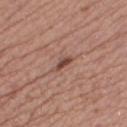Recorded during total-body skin imaging; not selected for excision or biopsy. Approximately 3 mm at its widest. A female patient, aged 38–42. Located on the right lower leg. Automated image analysis of the tile measured an eccentricity of roughly 0.9 and two-axis asymmetry of about 0.45. It also reported a border-irregularity index near 4.5/10, a within-lesion color-variation index near 0/10, and a peripheral color-asymmetry measure near 0. The software also gave a classifier nevus-likeness of about 25/100 and a detector confidence of about 100 out of 100 that the crop contains a lesion. This image is a 15 mm lesion crop taken from a total-body photograph.